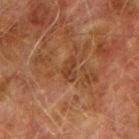The lesion was photographed on a routine skin check and not biopsied; there is no pathology result.